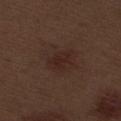Q: What kind of image is this?
A: total-body-photography crop, ~15 mm field of view
Q: What is the anatomic site?
A: the right thigh
Q: How was the tile lit?
A: white-light
Q: What is the lesion's diameter?
A: ~3.5 mm (longest diameter)
Q: Who is the patient?
A: male, aged 68 to 72